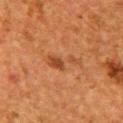The lesion was tiled from a total-body skin photograph and was not biopsied.
Imaged with cross-polarized lighting.
Longest diameter approximately 4.5 mm.
The patient is a female about 50 years old.
A 15 mm crop from a total-body photograph taken for skin-cancer surveillance.
From the right upper arm.
The total-body-photography lesion software estimated a footprint of about 6 mm², an eccentricity of roughly 0.95, and two-axis asymmetry of about 0.5. The software also gave a lesion color around L≈40 a*≈23 b*≈34 in CIELAB and about 7 CIELAB-L* units darker than the surrounding skin. The analysis additionally found a classifier nevus-likeness of about 35/100 and a lesion-detection confidence of about 100/100.